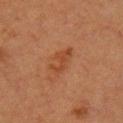workup — no biopsy performed (imaged during a skin exam)
illumination — cross-polarized illumination
diameter — ~4 mm (longest diameter)
TBP lesion metrics — an area of roughly 5 mm², a shape eccentricity near 0.85, and a shape-asymmetry score of about 0.5 (0 = symmetric); a mean CIELAB color near L≈35 a*≈21 b*≈30 and about 6 CIELAB-L* units darker than the surrounding skin; a color-variation rating of about 1/10 and radial color variation of about 0.5; a classifier nevus-likeness of about 5/100 and lesion-presence confidence of about 100/100
anatomic site — the chest
subject — male, roughly 50 years of age
image source — total-body-photography crop, ~15 mm field of view